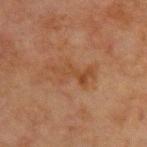Assessment: Imaged during a routine full-body skin examination; the lesion was not biopsied and no histopathology is available. Background: Imaged with cross-polarized lighting. Cropped from a total-body skin-imaging series; the visible field is about 15 mm. Automated image analysis of the tile measured an average lesion color of about L≈37 a*≈19 b*≈29 (CIELAB), roughly 5 lightness units darker than nearby skin, and a lesion-to-skin contrast of about 5.5 (normalized; higher = more distinct). It also reported a classifier nevus-likeness of about 0/100 and a lesion-detection confidence of about 100/100. The lesion is on the front of the torso. A male subject, about 65 years old.